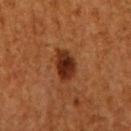The lesion was photographed on a routine skin check and not biopsied; there is no pathology result.